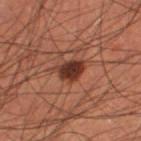  biopsy_status: not biopsied; imaged during a skin examination
  lighting: cross-polarized
  lesion_size:
    long_diameter_mm_approx: 3.5
  automated_metrics:
    area_mm2_approx: 6.5
    shape_asymmetry: 0.25
    border_irregularity_0_10: 2.5
    color_variation_0_10: 3.0
    peripheral_color_asymmetry: 1.0
    nevus_likeness_0_100: 90
    lesion_detection_confidence_0_100: 100
  site: right thigh
  patient:
    sex: male
    age_approx: 60
  image:
    source: total-body photography crop
    field_of_view_mm: 15